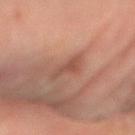The lesion was tiled from a total-body skin photograph and was not biopsied. On the right forearm. A roughly 15 mm field-of-view crop from a total-body skin photograph. A female patient, aged around 65. About 3 mm across. Imaged with cross-polarized lighting.